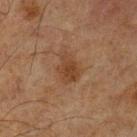Q: Was a biopsy performed?
A: total-body-photography surveillance lesion; no biopsy
Q: What is the anatomic site?
A: the leg
Q: How was the tile lit?
A: cross-polarized
Q: Patient demographics?
A: male, about 65 years old
Q: What did automated image analysis measure?
A: a footprint of about 7.5 mm², an eccentricity of roughly 0.7, and a symmetry-axis asymmetry near 0.3; a lesion–skin lightness drop of about 6 and a lesion-to-skin contrast of about 6.5 (normalized; higher = more distinct)
Q: How was this image acquired?
A: total-body-photography crop, ~15 mm field of view